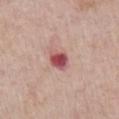  biopsy_status: not biopsied; imaged during a skin examination
  image:
    source: total-body photography crop
    field_of_view_mm: 15
  site: front of the torso
  patient:
    sex: male
    age_approx: 65
  lesion_size:
    long_diameter_mm_approx: 3.0